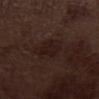Findings:
* workup · total-body-photography surveillance lesion; no biopsy
* TBP lesion metrics · a footprint of about 9.5 mm², an outline eccentricity of about 0.6 (0 = round, 1 = elongated), and a symmetry-axis asymmetry near 0.25; a border-irregularity rating of about 3/10, a color-variation rating of about 2.5/10, and radial color variation of about 1; a classifier nevus-likeness of about 5/100
* location · the abdomen
* image · ~15 mm tile from a whole-body skin photo
* lesion diameter · about 4 mm
* patient · male, aged around 70